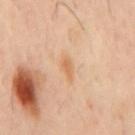The lesion was tiled from a total-body skin photograph and was not biopsied.